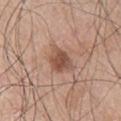| feature | finding |
|---|---|
| image source | total-body-photography crop, ~15 mm field of view |
| patient | male, aged 68 to 72 |
| tile lighting | white-light |
| site | the arm |
| automated metrics | a lesion area of about 7 mm²; a lesion color around L≈51 a*≈20 b*≈28 in CIELAB, roughly 11 lightness units darker than nearby skin, and a lesion-to-skin contrast of about 8 (normalized; higher = more distinct); a classifier nevus-likeness of about 60/100 and a lesion-detection confidence of about 100/100 |